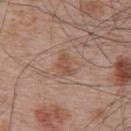No biopsy was performed on this lesion — it was imaged during a full skin examination and was not determined to be concerning. From the back. The lesion's longest dimension is about 2.5 mm. This is a white-light tile. The subject is a male aged around 65. This image is a 15 mm lesion crop taken from a total-body photograph.